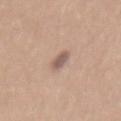The lesion was photographed on a routine skin check and not biopsied; there is no pathology result.
The lesion is on the mid back.
A roughly 15 mm field-of-view crop from a total-body skin photograph.
The lesion's longest dimension is about 3 mm.
The tile uses white-light illumination.
A female patient, roughly 35 years of age.